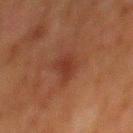Imaged during a routine full-body skin examination; the lesion was not biopsied and no histopathology is available. On the back. This image is a 15 mm lesion crop taken from a total-body photograph. This is a cross-polarized tile. The subject is a male aged 78–82.